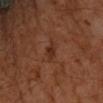Clinical impression:
This lesion was catalogued during total-body skin photography and was not selected for biopsy.
Context:
A 15 mm close-up tile from a total-body photography series done for melanoma screening. This is a cross-polarized tile. Measured at roughly 2.5 mm in maximum diameter. A male patient roughly 60 years of age. Automated image analysis of the tile measured a lesion color around L≈28 a*≈21 b*≈27 in CIELAB and roughly 6 lightness units darker than nearby skin. On the right forearm.